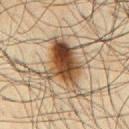| feature | finding |
|---|---|
| lighting | cross-polarized illumination |
| body site | the front of the torso |
| patient | male, in their mid-60s |
| lesion diameter | ~7 mm (longest diameter) |
| imaging modality | 15 mm crop, total-body photography |